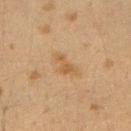Recorded during total-body skin imaging; not selected for excision or biopsy.
Captured under cross-polarized illumination.
The patient is a female aged 38–42.
Cropped from a total-body skin-imaging series; the visible field is about 15 mm.
Located on the right upper arm.
Approximately 3.5 mm at its widest.